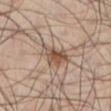Imaged during a routine full-body skin examination; the lesion was not biopsied and no histopathology is available. The total-body-photography lesion software estimated a lesion area of about 7.5 mm² and an eccentricity of roughly 0.8. The software also gave a lesion color around L≈50 a*≈16 b*≈26 in CIELAB, a lesion–skin lightness drop of about 11, and a normalized lesion–skin contrast near 8. The software also gave peripheral color asymmetry of about 2. A 15 mm close-up extracted from a 3D total-body photography capture. The lesion is located on the left lower leg. Captured under cross-polarized illumination. A male patient, in their mid-40s.